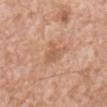lesion diameter: ≈3 mm | patient: male, roughly 60 years of age | automated metrics: a footprint of about 4.5 mm², a shape eccentricity near 0.6, and two-axis asymmetry of about 0.4; about 8 CIELAB-L* units darker than the surrounding skin and a lesion-to-skin contrast of about 5 (normalized; higher = more distinct); a detector confidence of about 100 out of 100 that the crop contains a lesion | image: 15 mm crop, total-body photography | location: the abdomen | illumination: white-light.